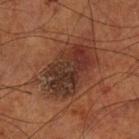Clinical impression:
Recorded during total-body skin imaging; not selected for excision or biopsy.
Image and clinical context:
Imaged with cross-polarized lighting. A close-up tile cropped from a whole-body skin photograph, about 15 mm across. The total-body-photography lesion software estimated an outline eccentricity of about 0.8 (0 = round, 1 = elongated). And it measured a lesion color around L≈33 a*≈20 b*≈26 in CIELAB and a normalized border contrast of about 9.5. The analysis additionally found a within-lesion color-variation index near 8.5/10 and peripheral color asymmetry of about 3. And it measured a classifier nevus-likeness of about 75/100 and a detector confidence of about 100 out of 100 that the crop contains a lesion. The subject is a male aged 68–72. On the left lower leg. The lesion's longest dimension is about 8.5 mm.